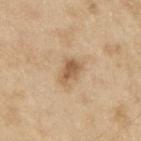Impression:
Recorded during total-body skin imaging; not selected for excision or biopsy.
Acquisition and patient details:
The lesion is on the right upper arm. A roughly 15 mm field-of-view crop from a total-body skin photograph. Approximately 3.5 mm at its widest. A male subject in their 70s. Captured under white-light illumination.